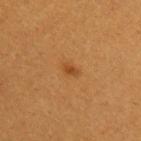Assessment:
Recorded during total-body skin imaging; not selected for excision or biopsy.
Clinical summary:
The recorded lesion diameter is about 1.5 mm. A female patient, in their 30s. The total-body-photography lesion software estimated an eccentricity of roughly 0.75. It also reported roughly 8 lightness units darker than nearby skin and a normalized border contrast of about 6.5. The analysis additionally found a detector confidence of about 100 out of 100 that the crop contains a lesion. A region of skin cropped from a whole-body photographic capture, roughly 15 mm wide. Located on the left upper arm. Imaged with cross-polarized lighting.